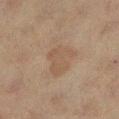Clinical impression:
Recorded during total-body skin imaging; not selected for excision or biopsy.
Background:
A 15 mm crop from a total-body photograph taken for skin-cancer surveillance. The tile uses cross-polarized illumination. A female patient, in their 40s. The lesion's longest dimension is about 4 mm. From the left lower leg. Automated image analysis of the tile measured a mean CIELAB color near L≈45 a*≈13 b*≈26, a lesion–skin lightness drop of about 5, and a normalized border contrast of about 5. The software also gave border irregularity of about 5 on a 0–10 scale, a color-variation rating of about 2/10, and peripheral color asymmetry of about 0.5.